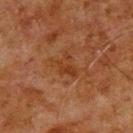Clinical summary: The lesion is on the upper back. Automated tile analysis of the lesion measured a mean CIELAB color near L≈30 a*≈20 b*≈29, roughly 5 lightness units darker than nearby skin, and a normalized border contrast of about 6. And it measured a border-irregularity rating of about 5.5/10, a within-lesion color-variation index near 2.5/10, and a peripheral color-asymmetry measure near 1. It also reported an automated nevus-likeness rating near 0 out of 100 and lesion-presence confidence of about 100/100. A roughly 15 mm field-of-view crop from a total-body skin photograph. Imaged with cross-polarized lighting. The subject is a male in their 80s. The lesion's longest dimension is about 3.5 mm.